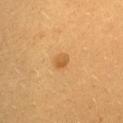Part of a total-body skin-imaging series; this lesion was reviewed on a skin check and was not flagged for biopsy. The lesion is located on the front of the torso. A 15 mm close-up tile from a total-body photography series done for melanoma screening. A female patient aged around 30.